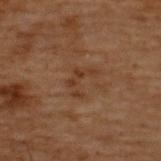Q: Is there a histopathology result?
A: no biopsy performed (imaged during a skin exam)
Q: Where on the body is the lesion?
A: the back
Q: How was this image acquired?
A: ~15 mm crop, total-body skin-cancer survey
Q: What are the patient's age and sex?
A: male, aged 68–72